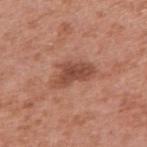Imaged during a routine full-body skin examination; the lesion was not biopsied and no histopathology is available. Automated tile analysis of the lesion measured a lesion area of about 10 mm², an eccentricity of roughly 0.85, and a shape-asymmetry score of about 0.4 (0 = symmetric). And it measured a mean CIELAB color near L≈49 a*≈25 b*≈29, about 11 CIELAB-L* units darker than the surrounding skin, and a lesion-to-skin contrast of about 8 (normalized; higher = more distinct). The software also gave an automated nevus-likeness rating near 75 out of 100. The tile uses white-light illumination. A 15 mm crop from a total-body photograph taken for skin-cancer surveillance. The lesion is located on the right upper arm. The patient is a female aged approximately 40. Approximately 5 mm at its widest.